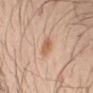<case>
<biopsy_status>not biopsied; imaged during a skin examination</biopsy_status>
<automated_metrics>
  <area_mm2_approx>4.0</area_mm2_approx>
  <eccentricity>0.75</eccentricity>
  <shape_asymmetry>0.2</shape_asymmetry>
  <vs_skin_darker_L>9.0</vs_skin_darker_L>
  <vs_skin_contrast_norm>7.0</vs_skin_contrast_norm>
  <border_irregularity_0_10>2.0</border_irregularity_0_10>
  <peripheral_color_asymmetry>1.0</peripheral_color_asymmetry>
  <nevus_likeness_0_100>80</nevus_likeness_0_100>
  <lesion_detection_confidence_0_100>100</lesion_detection_confidence_0_100>
</automated_metrics>
<lesion_size>
  <long_diameter_mm_approx>3.0</long_diameter_mm_approx>
</lesion_size>
<patient>
  <sex>male</sex>
  <age_approx>40</age_approx>
</patient>
<site>arm</site>
<image>
  <source>total-body photography crop</source>
  <field_of_view_mm>15</field_of_view_mm>
</image>
<lighting>cross-polarized</lighting>
</case>